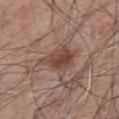workup: total-body-photography surveillance lesion; no biopsy | body site: the chest | lesion diameter: about 4.5 mm | image source: 15 mm crop, total-body photography | subject: male, approximately 65 years of age.